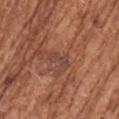notes = total-body-photography surveillance lesion; no biopsy | location = the left upper arm | illumination = white-light | acquisition = 15 mm crop, total-body photography | lesion size = about 3 mm | automated metrics = a lesion color around L≈43 a*≈22 b*≈26 in CIELAB and a lesion–skin lightness drop of about 6 | subject = female, aged around 75.